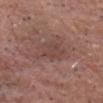biopsy_status: not biopsied; imaged during a skin examination
lighting: white-light
patient:
  sex: male
  age_approx: 80
image:
  source: total-body photography crop
  field_of_view_mm: 15
site: head or neck
automated_metrics:
  area_mm2_approx: 9.5
  eccentricity: 0.75
  shape_asymmetry: 0.3
  cielab_L: 44
  cielab_a: 19
  cielab_b: 23
  vs_skin_darker_L: 6.0
  vs_skin_contrast_norm: 4.5
  border_irregularity_0_10: 3.5
  color_variation_0_10: 2.5
  peripheral_color_asymmetry: 1.0
  nevus_likeness_0_100: 0
  lesion_detection_confidence_0_100: 100
lesion_size:
  long_diameter_mm_approx: 4.5